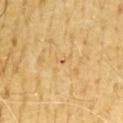The lesion was tiled from a total-body skin photograph and was not biopsied.
Approximately 1 mm at its widest.
An algorithmic analysis of the crop reported roughly 7 lightness units darker than nearby skin and a normalized lesion–skin contrast near 4.5. And it measured a border-irregularity index near 1.5/10 and peripheral color asymmetry of about 0.
Imaged with cross-polarized lighting.
On the chest.
A region of skin cropped from a whole-body photographic capture, roughly 15 mm wide.
The patient is a male about 65 years old.